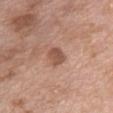| field | value |
|---|---|
| workup | total-body-photography surveillance lesion; no biopsy |
| tile lighting | white-light |
| acquisition | ~15 mm tile from a whole-body skin photo |
| patient | female, approximately 75 years of age |
| lesion size | ≈2.5 mm |
| body site | the chest |